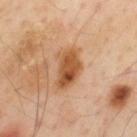imaging modality: ~15 mm crop, total-body skin-cancer survey
lighting: cross-polarized
site: the mid back
patient: male, in their mid-50s